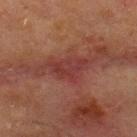biopsy status = catalogued during a skin exam; not biopsied
subject = male, aged 68–72
anatomic site = the back
acquisition = ~15 mm tile from a whole-body skin photo